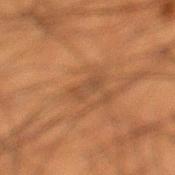Recorded during total-body skin imaging; not selected for excision or biopsy. Approximately 3.5 mm at its widest. An algorithmic analysis of the crop reported a lesion area of about 4 mm², an outline eccentricity of about 0.95 (0 = round, 1 = elongated), and a symmetry-axis asymmetry near 0.35. The analysis additionally found a lesion color around L≈37 a*≈17 b*≈28 in CIELAB, a lesion–skin lightness drop of about 6, and a normalized lesion–skin contrast near 5.5. It also reported internal color variation of about 2 on a 0–10 scale. Imaged with cross-polarized lighting. A 15 mm crop from a total-body photograph taken for skin-cancer surveillance. The subject is a male approximately 50 years of age. Located on the left lower leg.